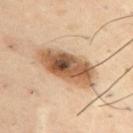Q: Lesion location?
A: the right upper arm
Q: What did automated image analysis measure?
A: a footprint of about 21 mm², an outline eccentricity of about 0.9 (0 = round, 1 = elongated), and a shape-asymmetry score of about 0.15 (0 = symmetric); a border-irregularity index near 2.5/10, internal color variation of about 10 on a 0–10 scale, and radial color variation of about 3.5
Q: Who is the patient?
A: male, aged 38 to 42
Q: What kind of image is this?
A: 15 mm crop, total-body photography
Q: How was the tile lit?
A: cross-polarized illumination